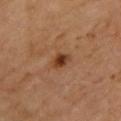<case>
<biopsy_status>not biopsied; imaged during a skin examination</biopsy_status>
<lesion_size>
  <long_diameter_mm_approx>2.5</long_diameter_mm_approx>
</lesion_size>
<lighting>cross-polarized</lighting>
<image>
  <source>total-body photography crop</source>
  <field_of_view_mm>15</field_of_view_mm>
</image>
<automated_metrics>
  <border_irregularity_0_10>2.5</border_irregularity_0_10>
  <color_variation_0_10>4.0</color_variation_0_10>
  <nevus_likeness_0_100>95</nevus_likeness_0_100>
</automated_metrics>
<site>upper back</site>
<patient>
  <sex>female</sex>
  <age_approx>70</age_approx>
</patient>
</case>